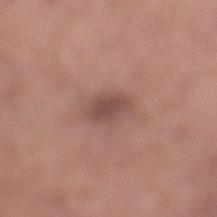Notes:
* notes: catalogued during a skin exam; not biopsied
* TBP lesion metrics: a border-irregularity rating of about 2/10, a color-variation rating of about 1.5/10, and peripheral color asymmetry of about 0.5
* location: the right lower leg
* patient: male, aged 43 to 47
* tile lighting: white-light illumination
* image: 15 mm crop, total-body photography